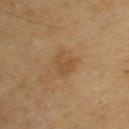Q: What kind of image is this?
A: ~15 mm crop, total-body skin-cancer survey
Q: Where on the body is the lesion?
A: the left upper arm
Q: How was the tile lit?
A: cross-polarized
Q: What are the patient's age and sex?
A: male, aged 53–57
Q: What is the lesion's diameter?
A: about 3 mm